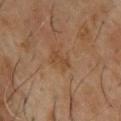The tile uses cross-polarized illumination. A lesion tile, about 15 mm wide, cut from a 3D total-body photograph. A male patient, in their mid- to late 60s. The lesion is located on the back.